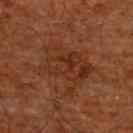<lesion>
<biopsy_status>not biopsied; imaged during a skin examination</biopsy_status>
</lesion>